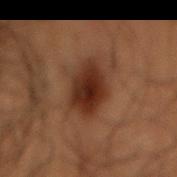The total-body-photography lesion software estimated a lesion area of about 13 mm² and a shape eccentricity near 0.85. This is a cross-polarized tile. On the lower back. Longest diameter approximately 6 mm. A male patient aged 48 to 52. Cropped from a total-body skin-imaging series; the visible field is about 15 mm.